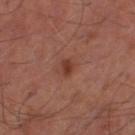| feature | finding |
|---|---|
| notes | imaged on a skin check; not biopsied |
| patient | male, in their mid-50s |
| automated metrics | a mean CIELAB color near L≈34 a*≈22 b*≈26, about 9 CIELAB-L* units darker than the surrounding skin, and a normalized lesion–skin contrast near 8; border irregularity of about 2 on a 0–10 scale and a peripheral color-asymmetry measure near 0.5; a lesion-detection confidence of about 100/100 |
| image | ~15 mm tile from a whole-body skin photo |
| illumination | cross-polarized |
| body site | the left thigh |